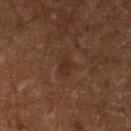Impression:
Part of a total-body skin-imaging series; this lesion was reviewed on a skin check and was not flagged for biopsy.
Image and clinical context:
The lesion is located on the left lower leg. Measured at roughly 2.5 mm in maximum diameter. This is a cross-polarized tile. A roughly 15 mm field-of-view crop from a total-body skin photograph. The lesion-visualizer software estimated a classifier nevus-likeness of about 0/100 and a lesion-detection confidence of about 100/100. A male subject aged 58–62.